From the left thigh. This image is a 15 mm lesion crop taken from a total-body photograph. A male patient, in their mid-50s: 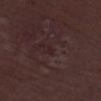biopsy diagnosis = a nodular basal cell carcinoma — a malignant skin lesion.